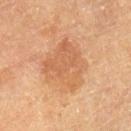<record>
<biopsy_status>not biopsied; imaged during a skin examination</biopsy_status>
<automated_metrics>
  <cielab_L>52</cielab_L>
  <cielab_a>21</cielab_a>
  <cielab_b>34</cielab_b>
  <vs_skin_darker_L>7.0</vs_skin_darker_L>
  <vs_skin_contrast_norm>5.5</vs_skin_contrast_norm>
  <border_irregularity_0_10>4.0</border_irregularity_0_10>
  <color_variation_0_10>3.5</color_variation_0_10>
  <nevus_likeness_0_100>15</nevus_likeness_0_100>
  <lesion_detection_confidence_0_100>100</lesion_detection_confidence_0_100>
</automated_metrics>
<image>
  <source>total-body photography crop</source>
  <field_of_view_mm>15</field_of_view_mm>
</image>
<patient>
  <sex>male</sex>
  <age_approx>85+</age_approx>
</patient>
<site>right lower leg</site>
<lighting>cross-polarized</lighting>
</record>